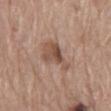The recorded lesion diameter is about 6 mm. From the mid back. This is a white-light tile. A lesion tile, about 15 mm wide, cut from a 3D total-body photograph. A male subject, in their 70s.